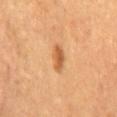Recorded during total-body skin imaging; not selected for excision or biopsy.
A female patient, aged 53 to 57.
Cropped from a whole-body photographic skin survey; the tile spans about 15 mm.
Located on the chest.